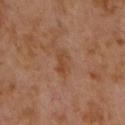Impression: Captured during whole-body skin photography for melanoma surveillance; the lesion was not biopsied. Context: The lesion is located on the front of the torso. A 15 mm close-up extracted from a 3D total-body photography capture. A male patient, approximately 60 years of age.